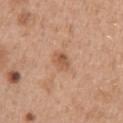No biopsy was performed on this lesion — it was imaged during a full skin examination and was not determined to be concerning. A 15 mm crop from a total-body photograph taken for skin-cancer surveillance. From the arm. A female patient aged 38–42.